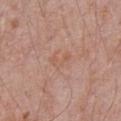Q: Was this lesion biopsied?
A: total-body-photography surveillance lesion; no biopsy
Q: What is the anatomic site?
A: the chest
Q: Who is the patient?
A: male, aged around 70
Q: Illumination type?
A: white-light illumination
Q: How was this image acquired?
A: ~15 mm tile from a whole-body skin photo
Q: Automated lesion metrics?
A: an area of roughly 3.5 mm², an outline eccentricity of about 0.85 (0 = round, 1 = elongated), and a shape-asymmetry score of about 0.6 (0 = symmetric); a mean CIELAB color near L≈57 a*≈21 b*≈30, roughly 5 lightness units darker than nearby skin, and a normalized lesion–skin contrast near 4.5; a color-variation rating of about 0/10 and radial color variation of about 0